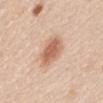The lesion was photographed on a routine skin check and not biopsied; there is no pathology result.
The lesion's longest dimension is about 4 mm.
The subject is a male approximately 45 years of age.
The lesion is located on the right upper arm.
A 15 mm crop from a total-body photograph taken for skin-cancer surveillance.
This is a white-light tile.